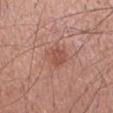Q: Was a biopsy performed?
A: catalogued during a skin exam; not biopsied
Q: How was this image acquired?
A: 15 mm crop, total-body photography
Q: Lesion location?
A: the left forearm
Q: How was the tile lit?
A: white-light
Q: Who is the patient?
A: male, approximately 35 years of age
Q: How large is the lesion?
A: about 3.5 mm